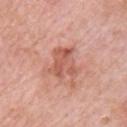Q: Was a biopsy performed?
A: total-body-photography surveillance lesion; no biopsy
Q: Who is the patient?
A: male, in their mid-50s
Q: Lesion size?
A: ~4.5 mm (longest diameter)
Q: Lesion location?
A: the chest
Q: How was this image acquired?
A: total-body-photography crop, ~15 mm field of view
Q: Automated lesion metrics?
A: a footprint of about 11 mm² and a shape eccentricity near 0.5; a mean CIELAB color near L≈58 a*≈26 b*≈30, roughly 10 lightness units darker than nearby skin, and a lesion-to-skin contrast of about 6.5 (normalized; higher = more distinct); a border-irregularity index near 4.5/10, a within-lesion color-variation index near 5/10, and radial color variation of about 1.5
Q: What lighting was used for the tile?
A: white-light